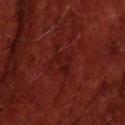* workup — total-body-photography surveillance lesion; no biopsy
* imaging modality — ~15 mm tile from a whole-body skin photo
* body site — the upper back
* patient — male, aged 68–72
* illumination — cross-polarized